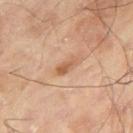Clinical impression: This lesion was catalogued during total-body skin photography and was not selected for biopsy. Clinical summary: Measured at roughly 3 mm in maximum diameter. A male patient, roughly 65 years of age. Captured under cross-polarized illumination. The lesion is located on the right thigh. Cropped from a whole-body photographic skin survey; the tile spans about 15 mm.